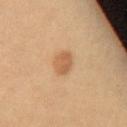biopsy status — no biopsy performed (imaged during a skin exam); patient — female, approximately 35 years of age; body site — the chest; image — ~15 mm crop, total-body skin-cancer survey.